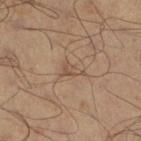* size: ≈3 mm
* lighting: cross-polarized
* body site: the left leg
* patient: male, roughly 50 years of age
* image: total-body-photography crop, ~15 mm field of view